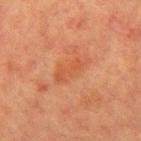Findings:
– workup — imaged on a skin check; not biopsied
– imaging modality — ~15 mm tile from a whole-body skin photo
– subject — female, aged 53–57
– body site — the right upper arm
– size — about 5 mm